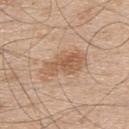* notes · total-body-photography surveillance lesion; no biopsy
* image source · ~15 mm tile from a whole-body skin photo
* lesion diameter · ≈6 mm
* tile lighting · white-light illumination
* patient · male, aged around 50
* site · the upper back